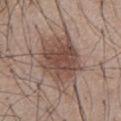Clinical impression: Imaged during a routine full-body skin examination; the lesion was not biopsied and no histopathology is available. Acquisition and patient details: From the chest. The lesion-visualizer software estimated a classifier nevus-likeness of about 85/100 and lesion-presence confidence of about 100/100. A male patient, roughly 55 years of age. A region of skin cropped from a whole-body photographic capture, roughly 15 mm wide.